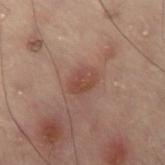Assessment: Recorded during total-body skin imaging; not selected for excision or biopsy.